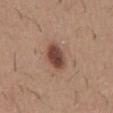Findings:
* acquisition: ~15 mm tile from a whole-body skin photo
* subject: male, aged approximately 60
* location: the front of the torso
* lighting: white-light
* automated lesion analysis: a lesion color around L≈44 a*≈21 b*≈26 in CIELAB, a lesion–skin lightness drop of about 15, and a normalized lesion–skin contrast near 11; a border-irregularity index near 2/10 and a peripheral color-asymmetry measure near 1.5